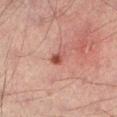Findings:
• workup — catalogued during a skin exam; not biopsied
• body site — the left lower leg
• tile lighting — cross-polarized
• image — 15 mm crop, total-body photography
• automated metrics — a footprint of about 2.5 mm² and a shape-asymmetry score of about 0.25 (0 = symmetric); a detector confidence of about 100 out of 100 that the crop contains a lesion
• patient — male, aged around 30